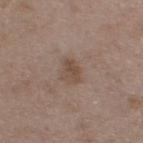This lesion was catalogued during total-body skin photography and was not selected for biopsy.
The lesion-visualizer software estimated an area of roughly 5 mm², an outline eccentricity of about 0.8 (0 = round, 1 = elongated), and a symmetry-axis asymmetry near 0.25. The software also gave border irregularity of about 2.5 on a 0–10 scale and peripheral color asymmetry of about 1.
On the left thigh.
The recorded lesion diameter is about 3 mm.
The subject is a male in their 50s.
A 15 mm crop from a total-body photograph taken for skin-cancer surveillance.